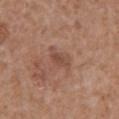Notes:
- follow-up — catalogued during a skin exam; not biopsied
- patient — female, aged approximately 70
- anatomic site — the chest
- illumination — white-light
- TBP lesion metrics — an area of roughly 5 mm², an outline eccentricity of about 0.85 (0 = round, 1 = elongated), and two-axis asymmetry of about 0.35; a lesion color around L≈48 a*≈21 b*≈28 in CIELAB, roughly 7 lightness units darker than nearby skin, and a normalized border contrast of about 5.5
- image — ~15 mm crop, total-body skin-cancer survey
- diameter — about 3.5 mm